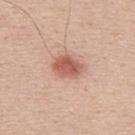workup: no biopsy performed (imaged during a skin exam) | location: the back | acquisition: ~15 mm tile from a whole-body skin photo | subject: male, about 40 years old.